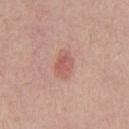This lesion was catalogued during total-body skin photography and was not selected for biopsy. Captured under white-light illumination. A region of skin cropped from a whole-body photographic capture, roughly 15 mm wide. The patient is a male roughly 40 years of age. On the chest. The total-body-photography lesion software estimated a lesion color around L≈57 a*≈25 b*≈26 in CIELAB. The software also gave a nevus-likeness score of about 90/100 and lesion-presence confidence of about 100/100. About 3 mm across.